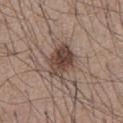notes=imaged on a skin check; not biopsied
image=total-body-photography crop, ~15 mm field of view
body site=the chest
image-analysis metrics=a footprint of about 11 mm² and two-axis asymmetry of about 0.2; an average lesion color of about L≈43 a*≈16 b*≈23 (CIELAB) and about 13 CIELAB-L* units darker than the surrounding skin; a classifier nevus-likeness of about 95/100 and a detector confidence of about 100 out of 100 that the crop contains a lesion
lesion diameter=≈4.5 mm
subject=male, in their mid-50s
tile lighting=white-light